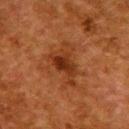Notes:
• workup · catalogued during a skin exam; not biopsied
• tile lighting · cross-polarized
• image source · ~15 mm crop, total-body skin-cancer survey
• automated metrics · an area of roughly 16 mm² and an eccentricity of roughly 0.7; roughly 7 lightness units darker than nearby skin and a normalized lesion–skin contrast near 7; an automated nevus-likeness rating near 45 out of 100
• lesion size · about 6 mm
• subject · female, aged 48–52
• site · the upper back